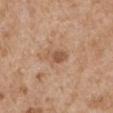| key | value |
|---|---|
| biopsy status | total-body-photography surveillance lesion; no biopsy |
| subject | male, approximately 65 years of age |
| lighting | white-light |
| diameter | ~3 mm (longest diameter) |
| image source | 15 mm crop, total-body photography |
| body site | the left upper arm |